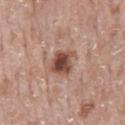Assessment: Recorded during total-body skin imaging; not selected for excision or biopsy. Context: A region of skin cropped from a whole-body photographic capture, roughly 15 mm wide. The lesion is on the lower back. The patient is a male aged around 70. Captured under white-light illumination. The lesion's longest dimension is about 3.5 mm.